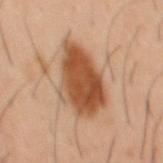An algorithmic analysis of the crop reported border irregularity of about 2.5 on a 0–10 scale, a within-lesion color-variation index near 5/10, and a peripheral color-asymmetry measure near 1.5. The analysis additionally found an automated nevus-likeness rating near 100 out of 100. A 15 mm crop from a total-body photograph taken for skin-cancer surveillance. On the mid back. Imaged with cross-polarized lighting. A male patient about 40 years old. The lesion's longest dimension is about 7.5 mm.